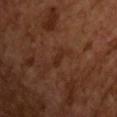Q: Was a biopsy performed?
A: catalogued during a skin exam; not biopsied
Q: What did automated image analysis measure?
A: a footprint of about 2 mm², an eccentricity of roughly 0.95, and two-axis asymmetry of about 0.3; an automated nevus-likeness rating near 0 out of 100 and lesion-presence confidence of about 95/100
Q: What kind of image is this?
A: 15 mm crop, total-body photography
Q: Lesion size?
A: ~2.5 mm (longest diameter)
Q: Illumination type?
A: cross-polarized illumination
Q: What are the patient's age and sex?
A: male, in their mid-60s